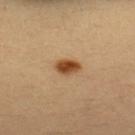  biopsy_status: not biopsied; imaged during a skin examination
  patient:
    sex: female
    age_approx: 35
  image:
    source: total-body photography crop
    field_of_view_mm: 15
  lesion_size:
    long_diameter_mm_approx: 3.0
  site: back
  automated_metrics:
    cielab_L: 48
    cielab_a: 22
    cielab_b: 38
    vs_skin_darker_L: 15.0
    border_irregularity_0_10: 1.5
    peripheral_color_asymmetry: 1.0